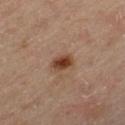follow-up: total-body-photography surveillance lesion; no biopsy
lesion diameter: ≈2.5 mm
image source: ~15 mm tile from a whole-body skin photo
patient: male, aged around 65
image-analysis metrics: an area of roughly 4.5 mm², a shape eccentricity near 0.7, and a symmetry-axis asymmetry near 0.2; a border-irregularity index near 1.5/10, internal color variation of about 4 on a 0–10 scale, and radial color variation of about 1
illumination: cross-polarized illumination
body site: the left thigh